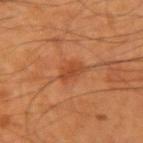<tbp_lesion>
<biopsy_status>not biopsied; imaged during a skin examination</biopsy_status>
<image>
  <source>total-body photography crop</source>
  <field_of_view_mm>15</field_of_view_mm>
</image>
<patient>
  <sex>male</sex>
  <age_approx>55</age_approx>
</patient>
<lesion_size>
  <long_diameter_mm_approx>2.5</long_diameter_mm_approx>
</lesion_size>
<lighting>cross-polarized</lighting>
<site>arm</site>
<automated_metrics>
  <area_mm2_approx>3.0</area_mm2_approx>
  <eccentricity>0.85</eccentricity>
  <shape_asymmetry>0.35</shape_asymmetry>
  <cielab_L>42</cielab_L>
  <cielab_a>27</cielab_a>
  <cielab_b>36</cielab_b>
  <vs_skin_darker_L>8.0</vs_skin_darker_L>
  <vs_skin_contrast_norm>6.0</vs_skin_contrast_norm>
</automated_metrics>
</tbp_lesion>